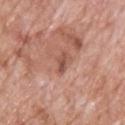Impression:
No biopsy was performed on this lesion — it was imaged during a full skin examination and was not determined to be concerning.
Image and clinical context:
A lesion tile, about 15 mm wide, cut from a 3D total-body photograph. About 2.5 mm across. An algorithmic analysis of the crop reported an eccentricity of roughly 0.9 and a shape-asymmetry score of about 0.3 (0 = symmetric). It also reported a color-variation rating of about 3/10 and peripheral color asymmetry of about 1. The analysis additionally found a nevus-likeness score of about 0/100 and a detector confidence of about 100 out of 100 that the crop contains a lesion. The tile uses white-light illumination. A male subject in their 70s. On the upper back.